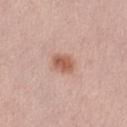tile lighting: white-light illumination | imaging modality: total-body-photography crop, ~15 mm field of view | body site: the left thigh | lesion size: ≈3 mm | subject: female, approximately 50 years of age.